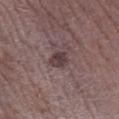{
  "biopsy_status": "not biopsied; imaged during a skin examination",
  "image": {
    "source": "total-body photography crop",
    "field_of_view_mm": 15
  },
  "patient": {
    "sex": "male",
    "age_approx": 75
  },
  "lighting": "white-light",
  "lesion_size": {
    "long_diameter_mm_approx": 2.5
  },
  "automated_metrics": {
    "area_mm2_approx": 4.0,
    "eccentricity": 0.6,
    "shape_asymmetry": 0.2,
    "color_variation_0_10": 2.5,
    "peripheral_color_asymmetry": 1.0
  },
  "site": "right lower leg"
}